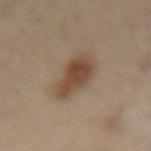The lesion was tiled from a total-body skin photograph and was not biopsied.
Automated tile analysis of the lesion measured a nevus-likeness score of about 100/100 and a lesion-detection confidence of about 100/100.
Imaged with cross-polarized lighting.
Located on the mid back.
Measured at roughly 5.5 mm in maximum diameter.
The patient is a female aged 58 to 62.
Cropped from a total-body skin-imaging series; the visible field is about 15 mm.